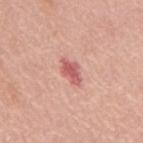Notes:
* patient — female, roughly 60 years of age
* acquisition — 15 mm crop, total-body photography
* TBP lesion metrics — a shape eccentricity near 0.85 and a symmetry-axis asymmetry near 0.25; a lesion color around L≈59 a*≈31 b*≈24 in CIELAB, about 12 CIELAB-L* units darker than the surrounding skin, and a lesion-to-skin contrast of about 8 (normalized; higher = more distinct); a within-lesion color-variation index near 2.5/10 and radial color variation of about 1; an automated nevus-likeness rating near 10 out of 100 and a detector confidence of about 100 out of 100 that the crop contains a lesion
* location — the mid back